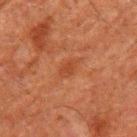Q: Was this lesion biopsied?
A: total-body-photography surveillance lesion; no biopsy
Q: How was this image acquired?
A: ~15 mm crop, total-body skin-cancer survey
Q: Patient demographics?
A: male, aged around 60
Q: What is the anatomic site?
A: the left upper arm